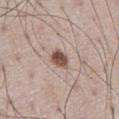biopsy status = no biopsy performed (imaged during a skin exam).